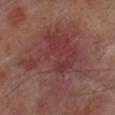subject: male, aged approximately 70
anatomic site: the right lower leg
lighting: cross-polarized
image source: total-body-photography crop, ~15 mm field of view